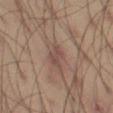Q: Is there a histopathology result?
A: catalogued during a skin exam; not biopsied
Q: What is the imaging modality?
A: ~15 mm crop, total-body skin-cancer survey
Q: Illumination type?
A: cross-polarized illumination
Q: What are the patient's age and sex?
A: male, aged 38 to 42
Q: What did automated image analysis measure?
A: a lesion area of about 4.5 mm², an eccentricity of roughly 0.8, and two-axis asymmetry of about 0.25; a mean CIELAB color near L≈47 a*≈18 b*≈23, a lesion–skin lightness drop of about 8, and a normalized lesion–skin contrast near 6; a border-irregularity rating of about 3/10 and peripheral color asymmetry of about 0.5
Q: What is the anatomic site?
A: the leg
Q: Lesion size?
A: ~3 mm (longest diameter)